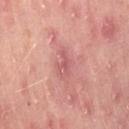Imaged during a routine full-body skin examination; the lesion was not biopsied and no histopathology is available.
From the abdomen.
A female subject, in their 50s.
A region of skin cropped from a whole-body photographic capture, roughly 15 mm wide.